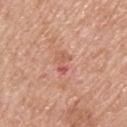Q: Was a biopsy performed?
A: no biopsy performed (imaged during a skin exam)
Q: Where on the body is the lesion?
A: the upper back
Q: How large is the lesion?
A: ≈2.5 mm
Q: What did automated image analysis measure?
A: a lesion area of about 2.5 mm² and a shape eccentricity near 0.85; a border-irregularity rating of about 5/10 and peripheral color asymmetry of about 0; an automated nevus-likeness rating near 0 out of 100 and a detector confidence of about 100 out of 100 that the crop contains a lesion
Q: What kind of image is this?
A: 15 mm crop, total-body photography
Q: What lighting was used for the tile?
A: white-light
Q: What are the patient's age and sex?
A: male, approximately 60 years of age